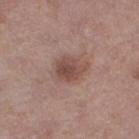| feature | finding |
|---|---|
| workup | imaged on a skin check; not biopsied |
| lesion diameter | about 4 mm |
| anatomic site | the right thigh |
| subject | female, about 50 years old |
| lighting | white-light illumination |
| image | ~15 mm tile from a whole-body skin photo |
| TBP lesion metrics | an outline eccentricity of about 0.7 (0 = round, 1 = elongated) and a symmetry-axis asymmetry near 0.25; roughly 10 lightness units darker than nearby skin |